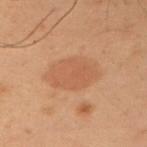follow-up = no biopsy performed (imaged during a skin exam) | site = the left upper arm | subject = male, aged 53 to 57 | illumination = cross-polarized | acquisition = 15 mm crop, total-body photography.